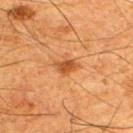The total-body-photography lesion software estimated a mean CIELAB color near L≈43 a*≈24 b*≈38, about 10 CIELAB-L* units darker than the surrounding skin, and a normalized lesion–skin contrast near 8. The analysis additionally found a border-irregularity rating of about 2.5/10, internal color variation of about 2 on a 0–10 scale, and peripheral color asymmetry of about 0.5. A 15 mm close-up extracted from a 3D total-body photography capture. Located on the upper back. This is a cross-polarized tile. A male subject aged 63 to 67. Approximately 2.5 mm at its widest.